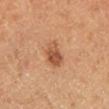follow-up = no biopsy performed (imaged during a skin exam)
illumination = cross-polarized illumination
subject = male, approximately 60 years of age
lesion diameter = ~3 mm (longest diameter)
imaging modality = ~15 mm crop, total-body skin-cancer survey
site = the left lower leg